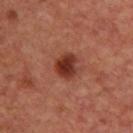This lesion was catalogued during total-body skin photography and was not selected for biopsy. The recorded lesion diameter is about 3 mm. A female subject aged around 45. The tile uses cross-polarized illumination. The lesion is located on the upper back. A roughly 15 mm field-of-view crop from a total-body skin photograph.